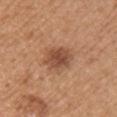Impression: Recorded during total-body skin imaging; not selected for excision or biopsy. Background: An algorithmic analysis of the crop reported an eccentricity of roughly 0.65 and two-axis asymmetry of about 0.2. The analysis additionally found a mean CIELAB color near L≈49 a*≈23 b*≈32 and a normalized border contrast of about 8. A female patient, roughly 55 years of age. Longest diameter approximately 4 mm. Captured under white-light illumination. A region of skin cropped from a whole-body photographic capture, roughly 15 mm wide. The lesion is located on the arm.